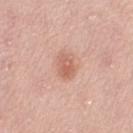Captured during whole-body skin photography for melanoma surveillance; the lesion was not biopsied.
Automated image analysis of the tile measured a footprint of about 6 mm² and an outline eccentricity of about 0.7 (0 = round, 1 = elongated). The analysis additionally found a lesion color around L≈62 a*≈24 b*≈29 in CIELAB, a lesion–skin lightness drop of about 9, and a normalized border contrast of about 6. The software also gave a border-irregularity index near 1.5/10, internal color variation of about 3.5 on a 0–10 scale, and peripheral color asymmetry of about 1.5. The software also gave a nevus-likeness score of about 40/100 and a lesion-detection confidence of about 100/100.
A female subject, in their 50s.
Approximately 3 mm at its widest.
The lesion is located on the abdomen.
The tile uses white-light illumination.
A 15 mm close-up tile from a total-body photography series done for melanoma screening.